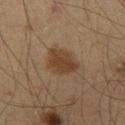notes: imaged on a skin check; not biopsied | patient: male, about 60 years old | acquisition: ~15 mm tile from a whole-body skin photo | site: the right thigh.